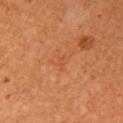notes: total-body-photography surveillance lesion; no biopsy | anatomic site: the left arm | patient: female, aged approximately 65 | illumination: cross-polarized illumination | acquisition: ~15 mm tile from a whole-body skin photo | diameter: about 1.5 mm | image-analysis metrics: an area of roughly 1 mm² and a shape-asymmetry score of about 0.45 (0 = symmetric); a lesion color around L≈50 a*≈30 b*≈38 in CIELAB, a lesion–skin lightness drop of about 4, and a lesion-to-skin contrast of about 3.5 (normalized; higher = more distinct); a border-irregularity rating of about 4/10, a color-variation rating of about 0/10, and radial color variation of about 0.